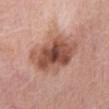A female patient aged 63 to 67. The recorded lesion diameter is about 7 mm. The tile uses white-light illumination. From the abdomen. A 15 mm close-up extracted from a 3D total-body photography capture.